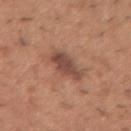Part of a total-body skin-imaging series; this lesion was reviewed on a skin check and was not flagged for biopsy.
Located on the arm.
A 15 mm crop from a total-body photograph taken for skin-cancer surveillance.
The lesion's longest dimension is about 4 mm.
Captured under white-light illumination.
A male subject aged approximately 40.